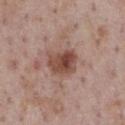image: ~15 mm crop, total-body skin-cancer survey | body site: the chest | patient: male, aged 73–77 | lighting: white-light illumination.